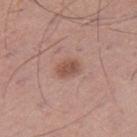Clinical impression:
The lesion was photographed on a routine skin check and not biopsied; there is no pathology result.
Image and clinical context:
Located on the leg. An algorithmic analysis of the crop reported an area of roughly 5.5 mm² and an eccentricity of roughly 0.65. The analysis additionally found an average lesion color of about L≈52 a*≈22 b*≈26 (CIELAB), roughly 10 lightness units darker than nearby skin, and a normalized lesion–skin contrast near 7. The analysis additionally found a border-irregularity index near 1.5/10, a within-lesion color-variation index near 2.5/10, and radial color variation of about 1. The lesion's longest dimension is about 3 mm. The tile uses white-light illumination. A 15 mm close-up extracted from a 3D total-body photography capture. The patient is a male aged 53–57.